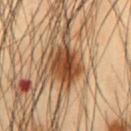Assessment:
Part of a total-body skin-imaging series; this lesion was reviewed on a skin check and was not flagged for biopsy.
Background:
The lesion is located on the abdomen. A 15 mm crop from a total-body photograph taken for skin-cancer surveillance. Measured at roughly 4 mm in maximum diameter. A male patient in their mid- to late 50s.